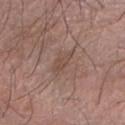workup = imaged on a skin check; not biopsied | automated lesion analysis = a shape eccentricity near 0.9 and two-axis asymmetry of about 0.3; a border-irregularity index near 3.5/10, a within-lesion color-variation index near 0.5/10, and peripheral color asymmetry of about 0; a nevus-likeness score of about 0/100 | body site = the left arm | subject = male, in their 70s | lesion size = ~3 mm (longest diameter) | lighting = white-light illumination | imaging modality = 15 mm crop, total-body photography.